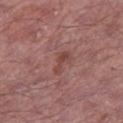Case summary:
• biopsy status · total-body-photography surveillance lesion; no biopsy
• tile lighting · white-light
• anatomic site · the right thigh
• image-analysis metrics · an average lesion color of about L≈44 a*≈23 b*≈23 (CIELAB), about 8 CIELAB-L* units darker than the surrounding skin, and a lesion-to-skin contrast of about 6.5 (normalized; higher = more distinct); internal color variation of about 0 on a 0–10 scale and radial color variation of about 0
• image source · ~15 mm tile from a whole-body skin photo
• patient · male, aged around 65
• diameter · about 3 mm